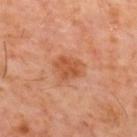The recorded lesion diameter is about 4 mm. Captured under cross-polarized illumination. From the back. Automated image analysis of the tile measured a classifier nevus-likeness of about 20/100 and a detector confidence of about 100 out of 100 that the crop contains a lesion. A male subject approximately 60 years of age. A region of skin cropped from a whole-body photographic capture, roughly 15 mm wide.